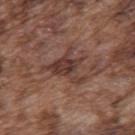Impression: No biopsy was performed on this lesion — it was imaged during a full skin examination and was not determined to be concerning. Acquisition and patient details: Approximately 4.5 mm at its widest. This image is a 15 mm lesion crop taken from a total-body photograph. On the upper back. The tile uses white-light illumination. Automated image analysis of the tile measured two-axis asymmetry of about 0.5. And it measured an average lesion color of about L≈37 a*≈19 b*≈23 (CIELAB), roughly 10 lightness units darker than nearby skin, and a lesion-to-skin contrast of about 8.5 (normalized; higher = more distinct). And it measured border irregularity of about 8.5 on a 0–10 scale, a within-lesion color-variation index near 3.5/10, and radial color variation of about 1. A male patient, in their mid- to late 70s.